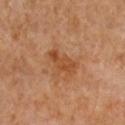Q: Is there a histopathology result?
A: catalogued during a skin exam; not biopsied